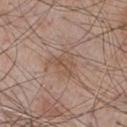workup = catalogued during a skin exam; not biopsied | automated metrics = a footprint of about 6.5 mm² and a shape eccentricity near 0.6; a mean CIELAB color near L≈52 a*≈17 b*≈27 and about 6 CIELAB-L* units darker than the surrounding skin; a classifier nevus-likeness of about 0/100 | site = the chest | illumination = white-light | acquisition = total-body-photography crop, ~15 mm field of view | subject = male, aged 63–67.